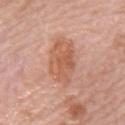workup=total-body-photography surveillance lesion; no biopsy
subject=female, aged approximately 65
acquisition=15 mm crop, total-body photography
lighting=white-light illumination
anatomic site=the right upper arm